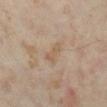| key | value |
|---|---|
| biopsy status | catalogued during a skin exam; not biopsied |
| image | 15 mm crop, total-body photography |
| body site | the right lower leg |
| patient | female, aged 33–37 |